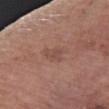Impression: Imaged during a routine full-body skin examination; the lesion was not biopsied and no histopathology is available. Image and clinical context: This is a white-light tile. A roughly 15 mm field-of-view crop from a total-body skin photograph. About 3 mm across. A male subject, roughly 60 years of age. From the right forearm.